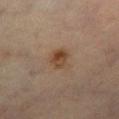Impression: The lesion was tiled from a total-body skin photograph and was not biopsied. Image and clinical context: A region of skin cropped from a whole-body photographic capture, roughly 15 mm wide. On the left lower leg. Imaged with cross-polarized lighting. The total-body-photography lesion software estimated an area of roughly 5.5 mm² and a shape eccentricity near 0.6. It also reported a mean CIELAB color near L≈38 a*≈15 b*≈27 and a normalized lesion–skin contrast near 8. The lesion's longest dimension is about 3 mm. The subject is a female aged approximately 70.